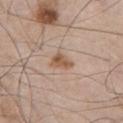workup: total-body-photography surveillance lesion; no biopsy
diameter: about 3 mm
site: the chest
lighting: white-light
acquisition: ~15 mm crop, total-body skin-cancer survey
patient: male, aged 53–57
image-analysis metrics: roughly 10 lightness units darker than nearby skin and a lesion-to-skin contrast of about 8 (normalized; higher = more distinct)